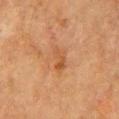notes=catalogued during a skin exam; not biopsied | tile lighting=cross-polarized | automated metrics=an area of roughly 4.5 mm², a shape eccentricity near 0.9, and two-axis asymmetry of about 0.4; a border-irregularity rating of about 4/10 and radial color variation of about 1 | acquisition=~15 mm tile from a whole-body skin photo | patient=female, aged 53 to 57 | lesion size=about 3.5 mm | body site=the chest.